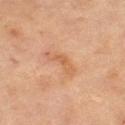Captured during whole-body skin photography for melanoma surveillance; the lesion was not biopsied.
An algorithmic analysis of the crop reported an area of roughly 4.5 mm² and two-axis asymmetry of about 0.65.
A 15 mm close-up tile from a total-body photography series done for melanoma screening.
Approximately 4 mm at its widest.
A female subject, aged around 70.
The tile uses cross-polarized illumination.
The lesion is located on the left thigh.